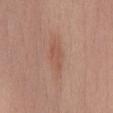- notes · no biopsy performed (imaged during a skin exam)
- site · the chest
- image source · 15 mm crop, total-body photography
- illumination · white-light illumination
- automated metrics · an area of roughly 5.5 mm²; an average lesion color of about L≈54 a*≈23 b*≈29 (CIELAB), about 6 CIELAB-L* units darker than the surrounding skin, and a lesion-to-skin contrast of about 5 (normalized; higher = more distinct)
- patient · female, aged approximately 60